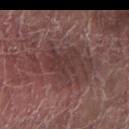No biopsy was performed on this lesion — it was imaged during a full skin examination and was not determined to be concerning. The lesion's longest dimension is about 9.5 mm. The subject is a male aged approximately 70. On the right forearm. Automated tile analysis of the lesion measured an automated nevus-likeness rating near 0 out of 100 and a lesion-detection confidence of about 90/100. A 15 mm close-up tile from a total-body photography series done for melanoma screening. The tile uses white-light illumination.